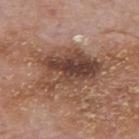Captured during whole-body skin photography for melanoma surveillance; the lesion was not biopsied.
About 7.5 mm across.
The subject is a male aged approximately 75.
The tile uses white-light illumination.
Cropped from a whole-body photographic skin survey; the tile spans about 15 mm.
From the upper back.
The total-body-photography lesion software estimated a border-irregularity index near 5/10, a color-variation rating of about 9/10, and a peripheral color-asymmetry measure near 3.5. The analysis additionally found a classifier nevus-likeness of about 5/100.